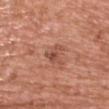Q: What is the anatomic site?
A: the chest
Q: What kind of image is this?
A: 15 mm crop, total-body photography
Q: Who is the patient?
A: male, in their mid-40s